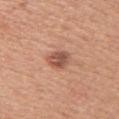  biopsy_status: not biopsied; imaged during a skin examination
  site: back
  lighting: white-light
  image:
    source: total-body photography crop
    field_of_view_mm: 15
  patient:
    sex: female
    age_approx: 45